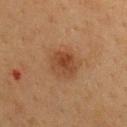The lesion was tiled from a total-body skin photograph and was not biopsied. Approximately 3 mm at its widest. A lesion tile, about 15 mm wide, cut from a 3D total-body photograph. Located on the upper back. Imaged with cross-polarized lighting. A female patient, in their 40s. The lesion-visualizer software estimated a footprint of about 7.5 mm², an eccentricity of roughly 0.45, and a shape-asymmetry score of about 0.2 (0 = symmetric). The analysis additionally found about 8 CIELAB-L* units darker than the surrounding skin. It also reported border irregularity of about 2 on a 0–10 scale, a color-variation rating of about 4/10, and a peripheral color-asymmetry measure near 1. The analysis additionally found a classifier nevus-likeness of about 75/100 and a lesion-detection confidence of about 100/100.